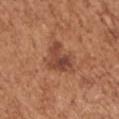Findings:
* workup — total-body-photography surveillance lesion; no biopsy
* lighting — white-light
* body site — the left upper arm
* automated lesion analysis — an area of roughly 9.5 mm², an eccentricity of roughly 0.75, and a symmetry-axis asymmetry near 0.45; a border-irregularity index near 5/10, internal color variation of about 6.5 on a 0–10 scale, and a peripheral color-asymmetry measure near 2.5
* image source — ~15 mm tile from a whole-body skin photo
* diameter — ≈4.5 mm
* subject — female, roughly 75 years of age